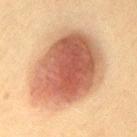biopsy status: catalogued during a skin exam; not biopsied
size: ≈9.5 mm
automated metrics: a lesion area of about 55 mm², a shape eccentricity near 0.65, and a shape-asymmetry score of about 0.15 (0 = symmetric); roughly 15 lightness units darker than nearby skin and a lesion-to-skin contrast of about 10.5 (normalized; higher = more distinct); a classifier nevus-likeness of about 100/100 and lesion-presence confidence of about 100/100
subject: female, aged approximately 50
tile lighting: cross-polarized illumination
image: total-body-photography crop, ~15 mm field of view
location: the left thigh